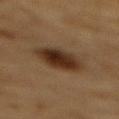biopsy status — no biopsy performed (imaged during a skin exam) | image — ~15 mm tile from a whole-body skin photo | anatomic site — the mid back | subject — male, in their mid-80s or older.